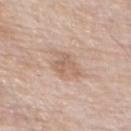<case>
<biopsy_status>not biopsied; imaged during a skin examination</biopsy_status>
<image>
  <source>total-body photography crop</source>
  <field_of_view_mm>15</field_of_view_mm>
</image>
<site>front of the torso</site>
<patient>
  <sex>male</sex>
  <age_approx>80</age_approx>
</patient>
<lesion_size>
  <long_diameter_mm_approx>3.5</long_diameter_mm_approx>
</lesion_size>
<lighting>white-light</lighting>
</case>